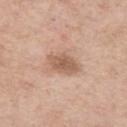Assessment:
Imaged during a routine full-body skin examination; the lesion was not biopsied and no histopathology is available.